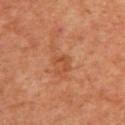Impression: The lesion was tiled from a total-body skin photograph and was not biopsied. Background: A female subject, approximately 40 years of age. An algorithmic analysis of the crop reported a lesion color around L≈50 a*≈28 b*≈38 in CIELAB, roughly 7 lightness units darker than nearby skin, and a lesion-to-skin contrast of about 5.5 (normalized; higher = more distinct). The software also gave border irregularity of about 6 on a 0–10 scale and internal color variation of about 0 on a 0–10 scale. From the upper back. Longest diameter approximately 2.5 mm. This image is a 15 mm lesion crop taken from a total-body photograph.